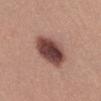follow-up: catalogued during a skin exam; not biopsied | imaging modality: ~15 mm crop, total-body skin-cancer survey | anatomic site: the back | automated lesion analysis: a border-irregularity index near 2/10, internal color variation of about 6.5 on a 0–10 scale, and radial color variation of about 2; a lesion-detection confidence of about 100/100 | diameter: about 5.5 mm | patient: female, aged 33 to 37.